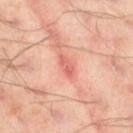Q: Is there a histopathology result?
A: no biopsy performed (imaged during a skin exam)
Q: Patient demographics?
A: male, aged around 45
Q: Automated lesion metrics?
A: an area of roughly 3 mm², an outline eccentricity of about 0.9 (0 = round, 1 = elongated), and two-axis asymmetry of about 0.35; border irregularity of about 3.5 on a 0–10 scale, a within-lesion color-variation index near 2.5/10, and radial color variation of about 0.5; a classifier nevus-likeness of about 0/100
Q: Where on the body is the lesion?
A: the left thigh
Q: What is the lesion's diameter?
A: about 3 mm
Q: How was this image acquired?
A: ~15 mm crop, total-body skin-cancer survey
Q: Illumination type?
A: cross-polarized